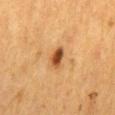Q: Was a biopsy performed?
A: no biopsy performed (imaged during a skin exam)
Q: Patient demographics?
A: female, aged 53 to 57
Q: How was this image acquired?
A: ~15 mm tile from a whole-body skin photo
Q: What is the anatomic site?
A: the mid back